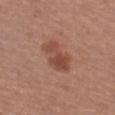* notes: no biopsy performed (imaged during a skin exam)
* tile lighting: white-light illumination
* TBP lesion metrics: a lesion area of about 9.5 mm², an outline eccentricity of about 0.85 (0 = round, 1 = elongated), and two-axis asymmetry of about 0.3; a mean CIELAB color near L≈47 a*≈23 b*≈29 and a lesion–skin lightness drop of about 9; internal color variation of about 4.5 on a 0–10 scale and radial color variation of about 1.5; a classifier nevus-likeness of about 15/100 and lesion-presence confidence of about 100/100
* site: the left thigh
* lesion diameter: ~4.5 mm (longest diameter)
* image source: 15 mm crop, total-body photography
* patient: female, aged approximately 55